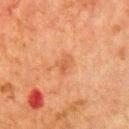follow-up: no biopsy performed (imaged during a skin exam); automated metrics: a border-irregularity index near 4/10, a within-lesion color-variation index near 0.5/10, and radial color variation of about 0; site: the chest; imaging modality: total-body-photography crop, ~15 mm field of view; lighting: cross-polarized illumination; patient: male, aged 78–82.